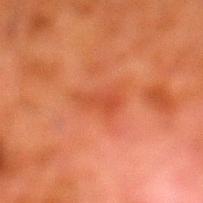Part of a total-body skin-imaging series; this lesion was reviewed on a skin check and was not flagged for biopsy.
A 15 mm close-up extracted from a 3D total-body photography capture.
The lesion is located on the left lower leg.
Measured at roughly 3.5 mm in maximum diameter.
The tile uses cross-polarized illumination.
An algorithmic analysis of the crop reported a lesion area of about 4 mm² and a shape-asymmetry score of about 0.45 (0 = symmetric). The analysis additionally found a mean CIELAB color near L≈40 a*≈30 b*≈35, about 6 CIELAB-L* units darker than the surrounding skin, and a lesion-to-skin contrast of about 5.5 (normalized; higher = more distinct). And it measured border irregularity of about 5 on a 0–10 scale.
A male subject aged 78 to 82.